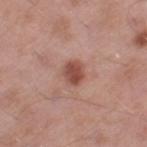Captured during whole-body skin photography for melanoma surveillance; the lesion was not biopsied.